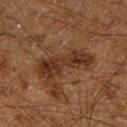{
  "biopsy_status": "not biopsied; imaged during a skin examination",
  "automated_metrics": {
    "vs_skin_darker_L": 8.0
  },
  "patient": {
    "sex": "male",
    "age_approx": 60
  },
  "image": {
    "source": "total-body photography crop",
    "field_of_view_mm": 15
  },
  "site": "right lower leg",
  "lesion_size": {
    "long_diameter_mm_approx": 6.5
  }
}